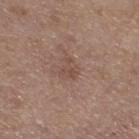Assessment: Captured during whole-body skin photography for melanoma surveillance; the lesion was not biopsied. Clinical summary: Captured under white-light illumination. On the right thigh. Longest diameter approximately 2.5 mm. A roughly 15 mm field-of-view crop from a total-body skin photograph. A female subject, about 65 years old.